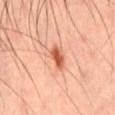biopsy_status: not biopsied; imaged during a skin examination
patient:
  sex: male
  age_approx: 45
site: front of the torso
image:
  source: total-body photography crop
  field_of_view_mm: 15